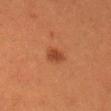Notes:
• notes · imaged on a skin check; not biopsied